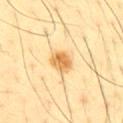follow-up — no biopsy performed (imaged during a skin exam)
TBP lesion metrics — a footprint of about 6 mm², a shape eccentricity near 0.45, and a shape-asymmetry score of about 0.15 (0 = symmetric); a color-variation rating of about 4.5/10 and radial color variation of about 1.5
subject — male, about 45 years old
lighting — cross-polarized illumination
image — ~15 mm tile from a whole-body skin photo
lesion diameter — about 3 mm
body site — the abdomen